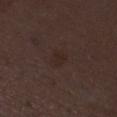biopsy status: total-body-photography surveillance lesion; no biopsy
lesion diameter: about 2.5 mm
site: the leg
lighting: white-light illumination
subject: female, approximately 50 years of age
imaging modality: 15 mm crop, total-body photography
automated metrics: border irregularity of about 2.5 on a 0–10 scale, internal color variation of about 2.5 on a 0–10 scale, and radial color variation of about 1